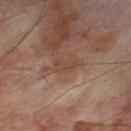Assessment:
Recorded during total-body skin imaging; not selected for excision or biopsy.
Acquisition and patient details:
On the left thigh. Automated image analysis of the tile measured an area of roughly 3 mm², a shape eccentricity near 0.85, and a symmetry-axis asymmetry near 0.4. The analysis additionally found border irregularity of about 4 on a 0–10 scale and peripheral color asymmetry of about 0. A close-up tile cropped from a whole-body skin photograph, about 15 mm across. Captured under cross-polarized illumination. Longest diameter approximately 2.5 mm. A male patient aged 68–72.